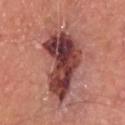workup = imaged on a skin check; not biopsied
size = ~8.5 mm (longest diameter)
image = ~15 mm tile from a whole-body skin photo
location = the head or neck
patient = male, aged 58–62
lighting = white-light illumination